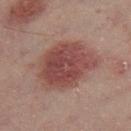{"biopsy_status": "not biopsied; imaged during a skin examination", "patient": {"sex": "male", "age_approx": 50}, "site": "right thigh", "image": {"source": "total-body photography crop", "field_of_view_mm": 15}, "lesion_size": {"long_diameter_mm_approx": 7.0}, "lighting": "cross-polarized"}